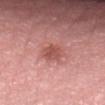The lesion was tiled from a total-body skin photograph and was not biopsied.
Imaged with white-light lighting.
The lesion's longest dimension is about 2.5 mm.
The subject is a female about 70 years old.
Cropped from a whole-body photographic skin survey; the tile spans about 15 mm.
The lesion is on the lower back.